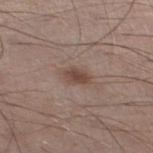Q: Was a biopsy performed?
A: total-body-photography surveillance lesion; no biopsy
Q: What did automated image analysis measure?
A: an eccentricity of roughly 0.8 and a shape-asymmetry score of about 0.2 (0 = symmetric); an average lesion color of about L≈45 a*≈16 b*≈24 (CIELAB) and a normalized lesion–skin contrast near 8; an automated nevus-likeness rating near 75 out of 100 and a lesion-detection confidence of about 100/100
Q: What are the patient's age and sex?
A: male, approximately 60 years of age
Q: What is the anatomic site?
A: the leg
Q: What is the lesion's diameter?
A: ~3 mm (longest diameter)
Q: What is the imaging modality?
A: ~15 mm tile from a whole-body skin photo